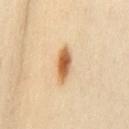<tbp_lesion>
  <biopsy_status>not biopsied; imaged during a skin examination</biopsy_status>
  <patient>
    <sex>female</sex>
    <age_approx>35</age_approx>
  </patient>
  <image>
    <source>total-body photography crop</source>
    <field_of_view_mm>15</field_of_view_mm>
  </image>
  <automated_metrics>
    <eccentricity>0.9</eccentricity>
    <shape_asymmetry>0.2</shape_asymmetry>
    <cielab_L>60</cielab_L>
    <cielab_a>20</cielab_a>
    <cielab_b>39</cielab_b>
    <vs_skin_darker_L>15.0</vs_skin_darker_L>
    <vs_skin_contrast_norm>10.0</vs_skin_contrast_norm>
    <border_irregularity_0_10>2.5</border_irregularity_0_10>
    <color_variation_0_10>5.0</color_variation_0_10>
    <peripheral_color_asymmetry>1.5</peripheral_color_asymmetry>
  </automated_metrics>
  <site>mid back</site>
  <lesion_size>
    <long_diameter_mm_approx>4.5</long_diameter_mm_approx>
  </lesion_size>
</tbp_lesion>